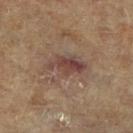Notes:
* imaging modality · 15 mm crop, total-body photography
* site · the right lower leg
* subject · male, in their mid-80s or older